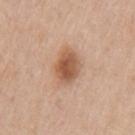Case summary:
- notes: total-body-photography surveillance lesion; no biopsy
- patient: male, aged approximately 60
- acquisition: ~15 mm crop, total-body skin-cancer survey
- lesion size: about 4 mm
- site: the left upper arm
- tile lighting: white-light illumination